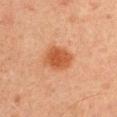Context: This is a cross-polarized tile. On the right upper arm. The recorded lesion diameter is about 3.5 mm. Automated image analysis of the tile measured a mean CIELAB color near L≈45 a*≈24 b*≈34, roughly 10 lightness units darker than nearby skin, and a normalized lesion–skin contrast near 8.5. The software also gave border irregularity of about 1.5 on a 0–10 scale, a within-lesion color-variation index near 2.5/10, and peripheral color asymmetry of about 0.5. The analysis additionally found a classifier nevus-likeness of about 100/100. A roughly 15 mm field-of-view crop from a total-body skin photograph. A male patient aged approximately 45.